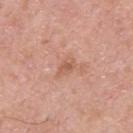Q: Is there a histopathology result?
A: imaged on a skin check; not biopsied